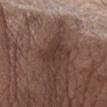notes: total-body-photography surveillance lesion; no biopsy | location: the abdomen | imaging modality: 15 mm crop, total-body photography | lighting: white-light illumination | lesion diameter: ~3.5 mm (longest diameter) | subject: male, aged approximately 75.